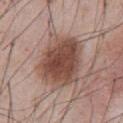follow-up — imaged on a skin check; not biopsied
size — ≈6.5 mm
acquisition — ~15 mm tile from a whole-body skin photo
anatomic site — the abdomen
patient — male, aged approximately 55